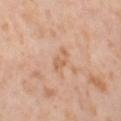Assessment:
Imaged during a routine full-body skin examination; the lesion was not biopsied and no histopathology is available.
Background:
Captured under cross-polarized illumination. The patient is a female approximately 55 years of age. The recorded lesion diameter is about 3 mm. A lesion tile, about 15 mm wide, cut from a 3D total-body photograph. The lesion-visualizer software estimated border irregularity of about 5 on a 0–10 scale, a color-variation rating of about 0.5/10, and radial color variation of about 0. The analysis additionally found a nevus-likeness score of about 0/100. Located on the leg.